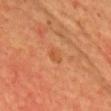Captured during whole-body skin photography for melanoma surveillance; the lesion was not biopsied. This is a cross-polarized tile. The subject is a male aged around 60. An algorithmic analysis of the crop reported a border-irregularity rating of about 3/10 and peripheral color asymmetry of about 1. And it measured a classifier nevus-likeness of about 0/100 and lesion-presence confidence of about 100/100. A 15 mm crop from a total-body photograph taken for skin-cancer surveillance. Approximately 2.5 mm at its widest.